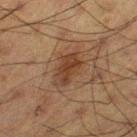site — the leg
subject — male, roughly 60 years of age
imaging modality — total-body-photography crop, ~15 mm field of view